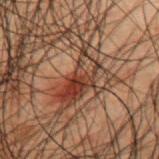notes — no biopsy performed (imaged during a skin exam)
image source — ~15 mm tile from a whole-body skin photo
subject — male, in their 50s
site — the upper back
lesion diameter — ~7 mm (longest diameter)
lighting — cross-polarized